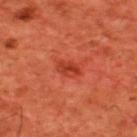Impression:
The lesion was tiled from a total-body skin photograph and was not biopsied.
Background:
The subject is a male aged 58 to 62. The lesion is located on the upper back. This image is a 15 mm lesion crop taken from a total-body photograph.